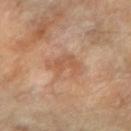The lesion is on the right forearm. The lesion's longest dimension is about 4.5 mm. A close-up tile cropped from a whole-body skin photograph, about 15 mm across. The lesion-visualizer software estimated a lesion color around L≈57 a*≈22 b*≈34 in CIELAB and roughly 8 lightness units darker than nearby skin. It also reported a nevus-likeness score of about 0/100. The patient is a female about 70 years old. Captured under cross-polarized illumination.